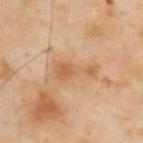Part of a total-body skin-imaging series; this lesion was reviewed on a skin check and was not flagged for biopsy.
This image is a 15 mm lesion crop taken from a total-body photograph.
From the upper back.
A male patient, in their mid- to late 50s.